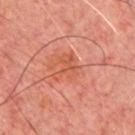The lesion was photographed on a routine skin check and not biopsied; there is no pathology result. The patient is a male about 45 years old. On the front of the torso. Automated tile analysis of the lesion measured border irregularity of about 7 on a 0–10 scale, a within-lesion color-variation index near 3/10, and peripheral color asymmetry of about 1. The analysis additionally found a detector confidence of about 100 out of 100 that the crop contains a lesion. A close-up tile cropped from a whole-body skin photograph, about 15 mm across. The lesion's longest dimension is about 3.5 mm.